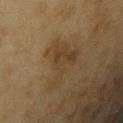follow-up = catalogued during a skin exam; not biopsied
image = total-body-photography crop, ~15 mm field of view
subject = female, about 55 years old
site = the right upper arm